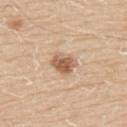Part of a total-body skin-imaging series; this lesion was reviewed on a skin check and was not flagged for biopsy. A 15 mm close-up tile from a total-body photography series done for melanoma screening. The tile uses white-light illumination. A male patient in their 60s. The lesion is on the back. The recorded lesion diameter is about 3 mm.